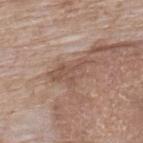  biopsy_status: not biopsied; imaged during a skin examination
  automated_metrics:
    cielab_L: 52
    cielab_a: 18
    cielab_b: 26
    vs_skin_darker_L: 8.0
    vs_skin_contrast_norm: 6.0
    color_variation_0_10: 2.5
    peripheral_color_asymmetry: 1.0
  image:
    source: total-body photography crop
    field_of_view_mm: 15
  patient:
    sex: female
    age_approx: 75
  site: upper back
  lesion_size:
    long_diameter_mm_approx: 5.5
  lighting: white-light